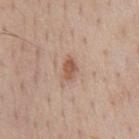{"biopsy_status": "not biopsied; imaged during a skin examination", "automated_metrics": {"area_mm2_approx": 3.5, "eccentricity": 0.8, "shape_asymmetry": 0.2, "cielab_L": 55, "cielab_a": 21, "cielab_b": 29, "vs_skin_darker_L": 11.0, "vs_skin_contrast_norm": 7.5}, "site": "chest", "image": {"source": "total-body photography crop", "field_of_view_mm": 15}, "patient": {"sex": "male", "age_approx": 60}, "lesion_size": {"long_diameter_mm_approx": 2.5}, "lighting": "white-light"}